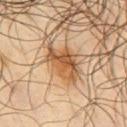workup = catalogued during a skin exam; not biopsied
location = the chest
diameter = ~5.5 mm (longest diameter)
patient = male, roughly 65 years of age
tile lighting = cross-polarized illumination
image source = total-body-photography crop, ~15 mm field of view